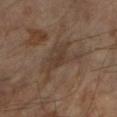notes = catalogued during a skin exam; not biopsied | body site = the right forearm | acquisition = 15 mm crop, total-body photography | automated lesion analysis = a footprint of about 15 mm²; a border-irregularity index near 7/10, internal color variation of about 2.5 on a 0–10 scale, and radial color variation of about 1 | lesion size = ~6.5 mm (longest diameter) | patient = male, in their mid- to late 60s.